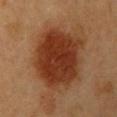Imaged during a routine full-body skin examination; the lesion was not biopsied and no histopathology is available.
Cropped from a total-body skin-imaging series; the visible field is about 15 mm.
The lesion is located on the upper back.
A female subject approximately 60 years of age.
The lesion's longest dimension is about 7 mm.
An algorithmic analysis of the crop reported an outline eccentricity of about 0.25 (0 = round, 1 = elongated) and two-axis asymmetry of about 0.15. The analysis additionally found border irregularity of about 2 on a 0–10 scale, internal color variation of about 4 on a 0–10 scale, and a peripheral color-asymmetry measure near 1.
The tile uses cross-polarized illumination.